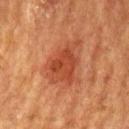Clinical impression: Part of a total-body skin-imaging series; this lesion was reviewed on a skin check and was not flagged for biopsy.